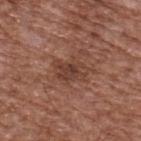Clinical impression: The lesion was photographed on a routine skin check and not biopsied; there is no pathology result. Clinical summary: About 3.5 mm across. From the back. The tile uses white-light illumination. Automated tile analysis of the lesion measured a footprint of about 7.5 mm², an outline eccentricity of about 0.8 (0 = round, 1 = elongated), and two-axis asymmetry of about 0.25. And it measured an average lesion color of about L≈40 a*≈21 b*≈26 (CIELAB), a lesion–skin lightness drop of about 8, and a lesion-to-skin contrast of about 6.5 (normalized; higher = more distinct). It also reported a border-irregularity index near 3/10. A male patient, aged around 75. A 15 mm close-up extracted from a 3D total-body photography capture.